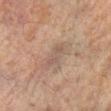The lesion was photographed on a routine skin check and not biopsied; there is no pathology result. A close-up tile cropped from a whole-body skin photograph, about 15 mm across. The lesion's longest dimension is about 3.5 mm. The patient is a male in their mid- to late 60s. The lesion is on the left forearm.